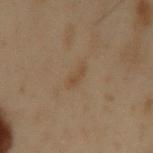The lesion was photographed on a routine skin check and not biopsied; there is no pathology result. A 15 mm crop from a total-body photograph taken for skin-cancer surveillance. A male subject, in their mid- to late 50s. On the left upper arm.